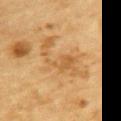<case>
<lesion_size>
  <long_diameter_mm_approx>7.0</long_diameter_mm_approx>
</lesion_size>
<image>
  <source>total-body photography crop</source>
  <field_of_view_mm>15</field_of_view_mm>
</image>
<site>upper back</site>
<patient>
  <sex>male</sex>
  <age_approx>85+</age_approx>
</patient>
<lighting>cross-polarized</lighting>
</case>